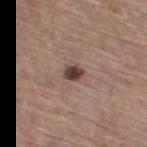* follow-up: imaged on a skin check; not biopsied
* imaging modality: ~15 mm tile from a whole-body skin photo
* patient: female, approximately 70 years of age
* lighting: white-light
* body site: the leg